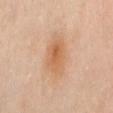<record>
  <biopsy_status>not biopsied; imaged during a skin examination</biopsy_status>
  <image>
    <source>total-body photography crop</source>
    <field_of_view_mm>15</field_of_view_mm>
  </image>
  <lesion_size>
    <long_diameter_mm_approx>5.5</long_diameter_mm_approx>
  </lesion_size>
  <lighting>cross-polarized</lighting>
  <site>mid back</site>
  <patient>
    <sex>female</sex>
    <age_approx>50</age_approx>
  </patient>
</record>